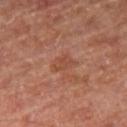workup = total-body-photography surveillance lesion; no biopsy
acquisition = ~15 mm crop, total-body skin-cancer survey
lighting = cross-polarized
image-analysis metrics = an average lesion color of about L≈45 a*≈25 b*≈30 (CIELAB), roughly 6 lightness units darker than nearby skin, and a normalized border contrast of about 5; a color-variation rating of about 1.5/10 and a peripheral color-asymmetry measure near 0.5; an automated nevus-likeness rating near 0 out of 100 and lesion-presence confidence of about 100/100
patient = male, aged around 70
diameter = ≈3 mm
body site = the right thigh